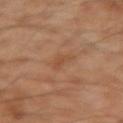This lesion was catalogued during total-body skin photography and was not selected for biopsy.
Approximately 3 mm at its widest.
Imaged with cross-polarized lighting.
A 15 mm close-up tile from a total-body photography series done for melanoma screening.
A male subject, in their mid-40s.
Automated tile analysis of the lesion measured a lesion area of about 4 mm², a shape eccentricity near 0.85, and a shape-asymmetry score of about 0.4 (0 = symmetric). It also reported a nevus-likeness score of about 0/100 and a detector confidence of about 100 out of 100 that the crop contains a lesion.
The lesion is located on the arm.